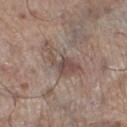Q: Who is the patient?
A: male, aged around 70
Q: Where on the body is the lesion?
A: the left lower leg
Q: What is the imaging modality?
A: ~15 mm crop, total-body skin-cancer survey
Q: What did automated image analysis measure?
A: a lesion area of about 8.5 mm² and an eccentricity of roughly 0.9; a classifier nevus-likeness of about 0/100 and lesion-presence confidence of about 85/100
Q: What lighting was used for the tile?
A: white-light